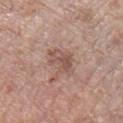<case>
  <biopsy_status>not biopsied; imaged during a skin examination</biopsy_status>
  <automated_metrics>
    <area_mm2_approx>7.5</area_mm2_approx>
    <eccentricity>0.8</eccentricity>
    <shape_asymmetry>0.35</shape_asymmetry>
    <nevus_likeness_0_100>5</nevus_likeness_0_100>
    <lesion_detection_confidence_0_100>100</lesion_detection_confidence_0_100>
  </automated_metrics>
  <image>
    <source>total-body photography crop</source>
    <field_of_view_mm>15</field_of_view_mm>
  </image>
  <patient>
    <sex>male</sex>
    <age_approx>55</age_approx>
  </patient>
  <lighting>white-light</lighting>
  <lesion_size>
    <long_diameter_mm_approx>4.5</long_diameter_mm_approx>
  </lesion_size>
</case>